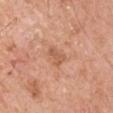Part of a total-body skin-imaging series; this lesion was reviewed on a skin check and was not flagged for biopsy.
On the left upper arm.
Cropped from a whole-body photographic skin survey; the tile spans about 15 mm.
A male patient, roughly 65 years of age.